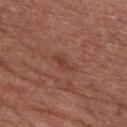Image and clinical context: The patient is a male roughly 60 years of age. The tile uses white-light illumination. A close-up tile cropped from a whole-body skin photograph, about 15 mm across. Located on the chest.